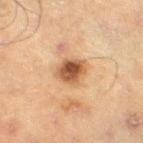notes = no biopsy performed (imaged during a skin exam).